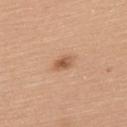Case summary:
* workup · imaged on a skin check; not biopsied
* lesion diameter · ~2.5 mm (longest diameter)
* site · the upper back
* imaging modality · 15 mm crop, total-body photography
* automated metrics · a border-irregularity rating of about 2/10 and a peripheral color-asymmetry measure near 1; a classifier nevus-likeness of about 90/100
* lighting · white-light illumination
* subject · female, aged around 65